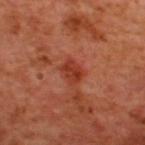Q: Patient demographics?
A: male, in their 50s
Q: Where on the body is the lesion?
A: the upper back
Q: What lighting was used for the tile?
A: cross-polarized
Q: How was this image acquired?
A: ~15 mm crop, total-body skin-cancer survey
Q: Automated lesion metrics?
A: a lesion area of about 5.5 mm², an outline eccentricity of about 0.65 (0 = round, 1 = elongated), and two-axis asymmetry of about 0.15; an automated nevus-likeness rating near 10 out of 100
Q: How large is the lesion?
A: about 2.5 mm